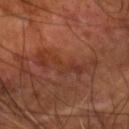follow-up: catalogued during a skin exam; not biopsied
image source: ~15 mm tile from a whole-body skin photo
subject: male, roughly 55 years of age
location: the right forearm